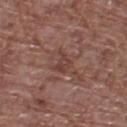The lesion was photographed on a routine skin check and not biopsied; there is no pathology result. The tile uses white-light illumination. The patient is a female aged approximately 75. From the left thigh. Cropped from a total-body skin-imaging series; the visible field is about 15 mm. Longest diameter approximately 3 mm.